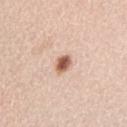Findings:
* biopsy status: catalogued during a skin exam; not biopsied
* subject: female, aged around 65
* lesion size: ≈2.5 mm
* image: total-body-photography crop, ~15 mm field of view
* site: the front of the torso